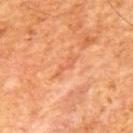Case summary:
* workup · catalogued during a skin exam; not biopsied
* lighting · cross-polarized
* lesion diameter · ~3 mm (longest diameter)
* image source · 15 mm crop, total-body photography
* patient · male, approximately 65 years of age
* automated metrics · a lesion area of about 2 mm², a shape eccentricity near 0.95, and two-axis asymmetry of about 0.55; a lesion color around L≈61 a*≈31 b*≈40 in CIELAB, about 7 CIELAB-L* units darker than the surrounding skin, and a normalized lesion–skin contrast near 4.5; a border-irregularity rating of about 7.5/10, internal color variation of about 0 on a 0–10 scale, and a peripheral color-asymmetry measure near 0